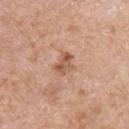Clinical impression: Imaged during a routine full-body skin examination; the lesion was not biopsied and no histopathology is available. Image and clinical context: Located on the chest. A 15 mm close-up extracted from a 3D total-body photography capture. Automated image analysis of the tile measured a footprint of about 4.5 mm², an outline eccentricity of about 0.7 (0 = round, 1 = elongated), and a symmetry-axis asymmetry near 0.3. The software also gave a mean CIELAB color near L≈56 a*≈23 b*≈31, roughly 10 lightness units darker than nearby skin, and a lesion-to-skin contrast of about 7 (normalized; higher = more distinct). The analysis additionally found peripheral color asymmetry of about 1.5. A male patient aged 68 to 72. Approximately 3 mm at its widest.